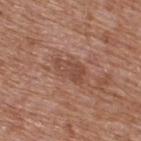follow-up: no biopsy performed (imaged during a skin exam) | tile lighting: white-light illumination | body site: the upper back | automated lesion analysis: an area of roughly 6.5 mm², a shape eccentricity near 0.85, and a shape-asymmetry score of about 0.3 (0 = symmetric); roughly 8 lightness units darker than nearby skin and a normalized lesion–skin contrast near 6; a classifier nevus-likeness of about 0/100 and a lesion-detection confidence of about 100/100 | patient: male, aged 63 to 67 | acquisition: ~15 mm tile from a whole-body skin photo | size: ~4 mm (longest diameter).